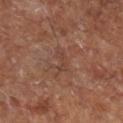Q: Was this lesion biopsied?
A: no biopsy performed (imaged during a skin exam)